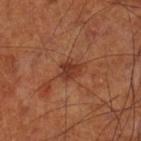Findings:
* workup · imaged on a skin check; not biopsied
* location · the left lower leg
* patient · male, aged 68–72
* image source · 15 mm crop, total-body photography
* size · ~3 mm (longest diameter)
* automated lesion analysis · a lesion area of about 4.5 mm²; a lesion color around L≈35 a*≈25 b*≈30 in CIELAB, about 9 CIELAB-L* units darker than the surrounding skin, and a normalized lesion–skin contrast near 8
* tile lighting · cross-polarized